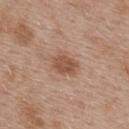Clinical impression: Recorded during total-body skin imaging; not selected for excision or biopsy.